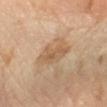<case>
<biopsy_status>not biopsied; imaged during a skin examination</biopsy_status>
<automated_metrics>
  <eccentricity>0.8</eccentricity>
  <shape_asymmetry>0.3</shape_asymmetry>
</automated_metrics>
<site>left forearm</site>
<image>
  <source>total-body photography crop</source>
  <field_of_view_mm>15</field_of_view_mm>
</image>
<lesion_size>
  <long_diameter_mm_approx>5.0</long_diameter_mm_approx>
</lesion_size>
<lighting>cross-polarized</lighting>
<patient>
  <sex>female</sex>
  <age_approx>60</age_approx>
</patient>
</case>